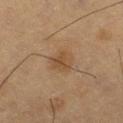Impression: Part of a total-body skin-imaging series; this lesion was reviewed on a skin check and was not flagged for biopsy.